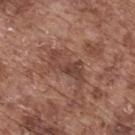{
  "biopsy_status": "not biopsied; imaged during a skin examination",
  "lighting": "white-light",
  "site": "back",
  "image": {
    "source": "total-body photography crop",
    "field_of_view_mm": 15
  },
  "lesion_size": {
    "long_diameter_mm_approx": 5.0
  },
  "patient": {
    "sex": "male",
    "age_approx": 75
  },
  "automated_metrics": {
    "area_mm2_approx": 8.0,
    "eccentricity": 0.9,
    "shape_asymmetry": 0.5,
    "cielab_L": 43,
    "cielab_a": 21,
    "cielab_b": 25,
    "vs_skin_darker_L": 8.0,
    "vs_skin_contrast_norm": 7.0
  }
}